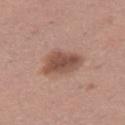illumination: white-light
subject: female, in their 30s
imaging modality: ~15 mm crop, total-body skin-cancer survey
automated metrics: a shape eccentricity near 0.75 and two-axis asymmetry of about 0.25; a lesion color around L≈50 a*≈21 b*≈26 in CIELAB, a lesion–skin lightness drop of about 12, and a normalized border contrast of about 8.5; a border-irregularity index near 2.5/10, a color-variation rating of about 3.5/10, and a peripheral color-asymmetry measure near 1; a classifier nevus-likeness of about 70/100 and lesion-presence confidence of about 100/100
body site: the right thigh